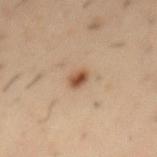The lesion was tiled from a total-body skin photograph and was not biopsied.
On the mid back.
Measured at roughly 2.5 mm in maximum diameter.
The patient is a male in their mid- to late 50s.
Cropped from a whole-body photographic skin survey; the tile spans about 15 mm.